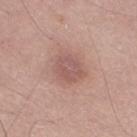Acquisition and patient details: A male subject, aged 48–52. This is a white-light tile. The recorded lesion diameter is about 3.5 mm. Cropped from a total-body skin-imaging series; the visible field is about 15 mm. The lesion is located on the leg.